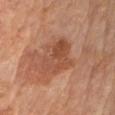Findings:
* biopsy status — no biopsy performed (imaged during a skin exam)
* automated metrics — a footprint of about 12 mm² and a shape eccentricity near 0.65; an average lesion color of about L≈46 a*≈23 b*≈30 (CIELAB); a nevus-likeness score of about 0/100
* size — ~4.5 mm (longest diameter)
* imaging modality — total-body-photography crop, ~15 mm field of view
* lighting — cross-polarized
* patient — male, in their mid-60s
* location — the left upper arm